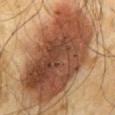The lesion was photographed on a routine skin check and not biopsied; there is no pathology result. This image is a 15 mm lesion crop taken from a total-body photograph. The recorded lesion diameter is about 11.5 mm. A male subject in their mid-60s. Automated image analysis of the tile measured an average lesion color of about L≈43 a*≈22 b*≈30 (CIELAB), a lesion–skin lightness drop of about 17, and a normalized border contrast of about 13. The analysis additionally found lesion-presence confidence of about 100/100. On the mid back. This is a cross-polarized tile.